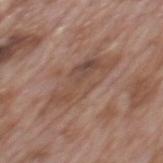site: the mid back; lighting: white-light illumination; lesion size: about 8.5 mm; subject: male, aged 68 to 72; image: total-body-photography crop, ~15 mm field of view.